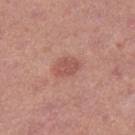Longest diameter approximately 3.5 mm.
Cropped from a total-body skin-imaging series; the visible field is about 15 mm.
An algorithmic analysis of the crop reported a shape eccentricity near 0.7 and a shape-asymmetry score of about 0.2 (0 = symmetric). It also reported an average lesion color of about L≈54 a*≈25 b*≈26 (CIELAB), about 8 CIELAB-L* units darker than the surrounding skin, and a normalized lesion–skin contrast near 6.
Captured under white-light illumination.
The lesion is located on the right thigh.
A female subject, aged 38 to 42.